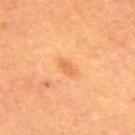Impression:
Captured during whole-body skin photography for melanoma surveillance; the lesion was not biopsied.
Context:
On the upper back. Approximately 2.5 mm at its widest. A 15 mm close-up extracted from a 3D total-body photography capture. Automated image analysis of the tile measured a normalized lesion–skin contrast near 5. It also reported a within-lesion color-variation index near 1/10 and peripheral color asymmetry of about 0.5. The software also gave a nevus-likeness score of about 35/100 and a lesion-detection confidence of about 100/100. Imaged with cross-polarized lighting. The patient is a male aged 48–52.